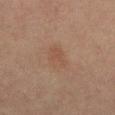workup — catalogued during a skin exam; not biopsied | anatomic site — the chest | image source — total-body-photography crop, ~15 mm field of view | image-analysis metrics — a mean CIELAB color near L≈39 a*≈15 b*≈24, a lesion–skin lightness drop of about 4, and a lesion-to-skin contrast of about 4.5 (normalized; higher = more distinct); border irregularity of about 3.5 on a 0–10 scale, a color-variation rating of about 1.5/10, and a peripheral color-asymmetry measure near 0.5 | lighting — cross-polarized | subject — male, aged 63–67.